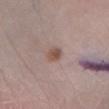notes = total-body-photography surveillance lesion; no biopsy
patient = female, aged around 70
site = the left lower leg
image = ~15 mm crop, total-body skin-cancer survey
TBP lesion metrics = a shape eccentricity near 0.6 and a symmetry-axis asymmetry near 0.25; a lesion color around L≈51 a*≈18 b*≈23 in CIELAB, a lesion–skin lightness drop of about 10, and a normalized lesion–skin contrast near 7.5; a border-irregularity rating of about 2/10 and a within-lesion color-variation index near 3/10; a lesion-detection confidence of about 100/100